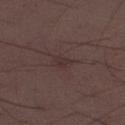Imaged during a routine full-body skin examination; the lesion was not biopsied and no histopathology is available. A male patient, roughly 30 years of age. The lesion is located on the left thigh. Cropped from a whole-body photographic skin survey; the tile spans about 15 mm.